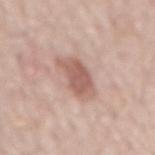<tbp_lesion>
  <biopsy_status>not biopsied; imaged during a skin examination</biopsy_status>
  <patient>
    <sex>male</sex>
    <age_approx>70</age_approx>
  </patient>
  <lighting>white-light</lighting>
  <image>
    <source>total-body photography crop</source>
    <field_of_view_mm>15</field_of_view_mm>
  </image>
  <site>mid back</site>
  <lesion_size>
    <long_diameter_mm_approx>5.0</long_diameter_mm_approx>
  </lesion_size>
  <automated_metrics>
    <area_mm2_approx>10.0</area_mm2_approx>
    <eccentricity>0.9</eccentricity>
  </automated_metrics>
</tbp_lesion>